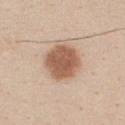Impression: Imaged during a routine full-body skin examination; the lesion was not biopsied and no histopathology is available. Image and clinical context: Cropped from a total-body skin-imaging series; the visible field is about 15 mm. The lesion's longest dimension is about 4.5 mm. The subject is a male roughly 35 years of age. The lesion is located on the chest. The lesion-visualizer software estimated a lesion–skin lightness drop of about 15 and a normalized lesion–skin contrast near 9.5. This is a white-light tile.